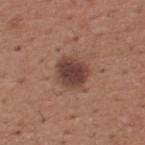workup: total-body-photography surveillance lesion; no biopsy | size: about 3.5 mm | body site: the upper back | lighting: white-light illumination | automated metrics: a lesion area of about 10 mm² and a shape eccentricity near 0.5 | subject: male, about 65 years old | imaging modality: ~15 mm crop, total-body skin-cancer survey.